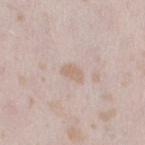biopsy status: total-body-photography surveillance lesion; no biopsy | body site: the left thigh | subject: female, aged 23–27 | image source: ~15 mm tile from a whole-body skin photo.